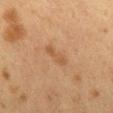Part of a total-body skin-imaging series; this lesion was reviewed on a skin check and was not flagged for biopsy.
A female subject, approximately 40 years of age.
The lesion is located on the mid back.
Automated tile analysis of the lesion measured an area of roughly 3.5 mm², an outline eccentricity of about 0.95 (0 = round, 1 = elongated), and a symmetry-axis asymmetry near 0.25. And it measured an average lesion color of about L≈44 a*≈18 b*≈32 (CIELAB), roughly 6 lightness units darker than nearby skin, and a normalized lesion–skin contrast near 5.5. And it measured border irregularity of about 4 on a 0–10 scale, a within-lesion color-variation index near 0.5/10, and radial color variation of about 0.
A 15 mm close-up extracted from a 3D total-body photography capture.
The recorded lesion diameter is about 4 mm.
This is a cross-polarized tile.